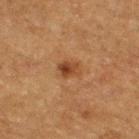<case>
<biopsy_status>not biopsied; imaged during a skin examination</biopsy_status>
<lesion_size>
  <long_diameter_mm_approx>3.0</long_diameter_mm_approx>
</lesion_size>
<image>
  <source>total-body photography crop</source>
  <field_of_view_mm>15</field_of_view_mm>
</image>
<site>right thigh</site>
<patient>
  <sex>male</sex>
  <age_approx>75</age_approx>
</patient>
<automated_metrics>
  <area_mm2_approx>4.5</area_mm2_approx>
  <eccentricity>0.7</eccentricity>
  <shape_asymmetry>0.3</shape_asymmetry>
  <nevus_likeness_0_100>85</nevus_likeness_0_100>
  <lesion_detection_confidence_0_100>100</lesion_detection_confidence_0_100>
</automated_metrics>
</case>